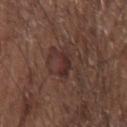{"biopsy_status": "not biopsied; imaged during a skin examination", "patient": {"sex": "male", "age_approx": 65}, "image": {"source": "total-body photography crop", "field_of_view_mm": 15}, "lighting": "white-light", "automated_metrics": {"cielab_L": 30, "cielab_a": 18, "cielab_b": 20, "vs_skin_darker_L": 7.0, "vs_skin_contrast_norm": 7.0, "border_irregularity_0_10": 6.5, "color_variation_0_10": 1.5, "peripheral_color_asymmetry": 0.5}, "site": "left forearm", "lesion_size": {"long_diameter_mm_approx": 3.5}}